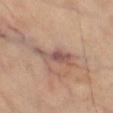Recorded during total-body skin imaging; not selected for excision or biopsy.
Longest diameter approximately 5 mm.
A male subject aged around 65.
On the left thigh.
A close-up tile cropped from a whole-body skin photograph, about 15 mm across.